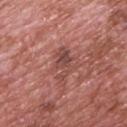The lesion was tiled from a total-body skin photograph and was not biopsied.
Cropped from a total-body skin-imaging series; the visible field is about 15 mm.
Measured at roughly 4 mm in maximum diameter.
A male patient approximately 70 years of age.
The lesion is located on the upper back.